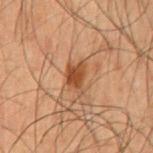This lesion was catalogued during total-body skin photography and was not selected for biopsy. The tile uses cross-polarized illumination. The lesion's longest dimension is about 3.5 mm. This image is a 15 mm lesion crop taken from a total-body photograph. From the abdomen. The patient is a male in their 50s.